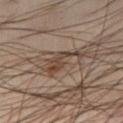Image and clinical context: This is a cross-polarized tile. Measured at roughly 5.5 mm in maximum diameter. A roughly 15 mm field-of-view crop from a total-body skin photograph. Automated tile analysis of the lesion measured an average lesion color of about L≈43 a*≈15 b*≈25 (CIELAB) and roughly 9 lightness units darker than nearby skin. It also reported an automated nevus-likeness rating near 5 out of 100 and a lesion-detection confidence of about 70/100. Located on the right thigh. The patient is a male aged 48 to 52.